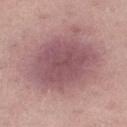Q: Was this lesion biopsied?
A: no biopsy performed (imaged during a skin exam)
Q: Where on the body is the lesion?
A: the right thigh
Q: Lesion size?
A: ~8 mm (longest diameter)
Q: What is the imaging modality?
A: total-body-photography crop, ~15 mm field of view
Q: Patient demographics?
A: female, in their mid-30s
Q: What did automated image analysis measure?
A: a lesion area of about 45 mm² and an outline eccentricity of about 0.45 (0 = round, 1 = elongated); a border-irregularity index near 2.5/10; a classifier nevus-likeness of about 10/100 and a detector confidence of about 100 out of 100 that the crop contains a lesion